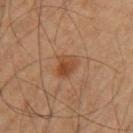Recorded during total-body skin imaging; not selected for excision or biopsy. On the right upper arm. A close-up tile cropped from a whole-body skin photograph, about 15 mm across. A male subject, aged approximately 60.